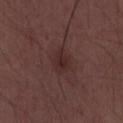Captured during whole-body skin photography for melanoma surveillance; the lesion was not biopsied.
Located on the abdomen.
This image is a 15 mm lesion crop taken from a total-body photograph.
A male patient, aged 28–32.